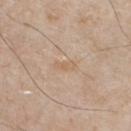{"biopsy_status": "not biopsied; imaged during a skin examination", "patient": {"sex": "male", "age_approx": 55}, "automated_metrics": {"cielab_L": 62, "cielab_a": 16, "cielab_b": 32, "vs_skin_contrast_norm": 5.0, "nevus_likeness_0_100": 0, "lesion_detection_confidence_0_100": 100}, "lesion_size": {"long_diameter_mm_approx": 2.5}, "image": {"source": "total-body photography crop", "field_of_view_mm": 15}, "site": "chest", "lighting": "white-light"}